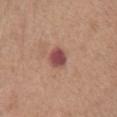Q: Was this lesion biopsied?
A: no biopsy performed (imaged during a skin exam)
Q: How was the tile lit?
A: white-light illumination
Q: Automated lesion metrics?
A: a mean CIELAB color near L≈48 a*≈27 b*≈23 and about 14 CIELAB-L* units darker than the surrounding skin; a border-irregularity index near 1.5/10 and a peripheral color-asymmetry measure near 1.5; lesion-presence confidence of about 100/100
Q: What is the anatomic site?
A: the abdomen
Q: How was this image acquired?
A: total-body-photography crop, ~15 mm field of view
Q: How large is the lesion?
A: about 2.5 mm
Q: What are the patient's age and sex?
A: female, about 75 years old